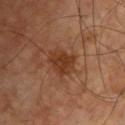Longest diameter approximately 3.5 mm.
This is a cross-polarized tile.
The lesion is on the chest.
A roughly 15 mm field-of-view crop from a total-body skin photograph.
The subject is a male aged around 60.